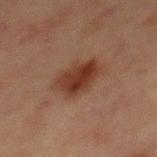Part of a total-body skin-imaging series; this lesion was reviewed on a skin check and was not flagged for biopsy.
Cropped from a total-body skin-imaging series; the visible field is about 15 mm.
A male patient, aged approximately 65.
From the abdomen.